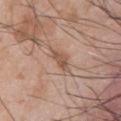{"biopsy_status": "not biopsied; imaged during a skin examination", "image": {"source": "total-body photography crop", "field_of_view_mm": 15}, "site": "front of the torso", "lighting": "white-light", "patient": {"sex": "male", "age_approx": 50}}